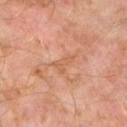  biopsy_status: not biopsied; imaged during a skin examination
  patient:
    sex: male
    age_approx: 60
  lesion_size:
    long_diameter_mm_approx: 3.0
  site: left lower leg
  image:
    source: total-body photography crop
    field_of_view_mm: 15